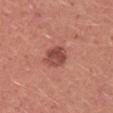The subject is a male about 25 years old. This is a white-light tile. Longest diameter approximately 3 mm. On the right upper arm. This image is a 15 mm lesion crop taken from a total-body photograph.